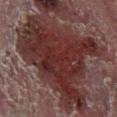Q: Was a biopsy performed?
A: no biopsy performed (imaged during a skin exam)
Q: Lesion size?
A: about 13.5 mm
Q: How was the tile lit?
A: cross-polarized illumination
Q: How was this image acquired?
A: ~15 mm crop, total-body skin-cancer survey
Q: Automated lesion metrics?
A: a mean CIELAB color near L≈26 a*≈20 b*≈18 and roughly 11 lightness units darker than nearby skin; a border-irregularity rating of about 7/10, a color-variation rating of about 5.5/10, and radial color variation of about 2; a nevus-likeness score of about 0/100 and lesion-presence confidence of about 65/100
Q: Who is the patient?
A: male, approximately 55 years of age
Q: What is the anatomic site?
A: the left lower leg